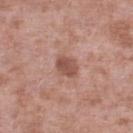Impression:
Imaged during a routine full-body skin examination; the lesion was not biopsied and no histopathology is available.
Context:
Approximately 3 mm at its widest. A male patient, about 55 years old. A close-up tile cropped from a whole-body skin photograph, about 15 mm across. Located on the left lower leg. Captured under white-light illumination.